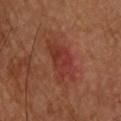<case>
  <biopsy_status>not biopsied; imaged during a skin examination</biopsy_status>
  <lighting>cross-polarized</lighting>
  <site>upper back</site>
  <lesion_size>
    <long_diameter_mm_approx>5.5</long_diameter_mm_approx>
  </lesion_size>
  <patient>
    <sex>male</sex>
    <age_approx>60</age_approx>
  </patient>
  <image>
    <source>total-body photography crop</source>
    <field_of_view_mm>15</field_of_view_mm>
  </image>
</case>